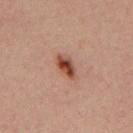Part of a total-body skin-imaging series; this lesion was reviewed on a skin check and was not flagged for biopsy. The patient is a male aged around 30. Approximately 3.5 mm at its widest. Captured under cross-polarized illumination. The lesion is located on the back. Cropped from a whole-body photographic skin survey; the tile spans about 15 mm.